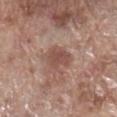Notes:
- biopsy status: imaged on a skin check; not biopsied
- acquisition: ~15 mm tile from a whole-body skin photo
- illumination: white-light illumination
- subject: male, aged 68–72
- lesion diameter: ~3.5 mm (longest diameter)
- body site: the right lower leg
- automated lesion analysis: a lesion area of about 7 mm² and an outline eccentricity of about 0.65 (0 = round, 1 = elongated); a border-irregularity rating of about 3/10, internal color variation of about 2 on a 0–10 scale, and a peripheral color-asymmetry measure near 0.5